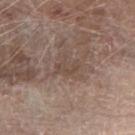The lesion was tiled from a total-body skin photograph and was not biopsied. About 3 mm across. A 15 mm crop from a total-body photograph taken for skin-cancer surveillance. Automated tile analysis of the lesion measured a lesion color around L≈46 a*≈15 b*≈22 in CIELAB, roughly 6 lightness units darker than nearby skin, and a normalized lesion–skin contrast near 4.5. The software also gave an automated nevus-likeness rating near 0 out of 100 and lesion-presence confidence of about 75/100. The patient is a male aged 78 to 82. From the left forearm. The tile uses white-light illumination.